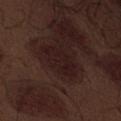image:
  source: total-body photography crop
  field_of_view_mm: 15
patient:
  sex: male
  age_approx: 70
lesion_size:
  long_diameter_mm_approx: 5.5
automated_metrics:
  area_mm2_approx: 10.0
  eccentricity: 0.85
  shape_asymmetry: 0.25
  vs_skin_darker_L: 5.0
  lesion_detection_confidence_0_100: 85
site: front of the torso
lighting: white-light